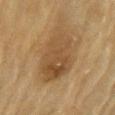tile lighting: cross-polarized | image-analysis metrics: a lesion color around L≈42 a*≈14 b*≈31 in CIELAB, roughly 9 lightness units darker than nearby skin, and a normalized lesion–skin contrast near 7; a nevus-likeness score of about 10/100 and a detector confidence of about 100 out of 100 that the crop contains a lesion | patient: male, aged 83 or older | location: the left upper arm | imaging modality: 15 mm crop, total-body photography | lesion size: ≈8 mm.